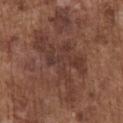Q: What lighting was used for the tile?
A: white-light illumination
Q: Where on the body is the lesion?
A: the chest
Q: Patient demographics?
A: male, aged approximately 75
Q: What kind of image is this?
A: 15 mm crop, total-body photography